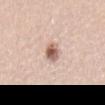Impression: No biopsy was performed on this lesion — it was imaged during a full skin examination and was not determined to be concerning. Clinical summary: Automated tile analysis of the lesion measured a lesion color around L≈60 a*≈19 b*≈26 in CIELAB, roughly 16 lightness units darker than nearby skin, and a normalized lesion–skin contrast near 9.5. The lesion's longest dimension is about 2.5 mm. On the lower back. Captured under white-light illumination. A region of skin cropped from a whole-body photographic capture, roughly 15 mm wide. A female subject, about 45 years old.